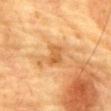workup: no biopsy performed (imaged during a skin exam)
image source: ~15 mm tile from a whole-body skin photo
lesion size: ~2.5 mm (longest diameter)
body site: the chest
lighting: cross-polarized illumination
subject: male, roughly 85 years of age or older